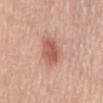Q: What are the patient's age and sex?
A: female, aged around 65
Q: Lesion location?
A: the mid back
Q: What kind of image is this?
A: 15 mm crop, total-body photography
Q: How large is the lesion?
A: about 3.5 mm